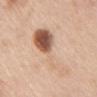Q: Was this lesion biopsied?
A: catalogued during a skin exam; not biopsied
Q: How was this image acquired?
A: ~15 mm tile from a whole-body skin photo
Q: How large is the lesion?
A: about 9.5 mm
Q: Patient demographics?
A: female, aged approximately 50
Q: Where on the body is the lesion?
A: the chest
Q: What did automated image analysis measure?
A: a footprint of about 20 mm², an eccentricity of roughly 0.95, and a shape-asymmetry score of about 0.6 (0 = symmetric); an automated nevus-likeness rating near 100 out of 100 and lesion-presence confidence of about 100/100
Q: Illumination type?
A: white-light illumination